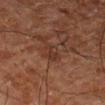Recorded during total-body skin imaging; not selected for excision or biopsy. A male subject aged 78 to 82. Captured under cross-polarized illumination. The lesion is located on the left thigh. The recorded lesion diameter is about 2.5 mm. An algorithmic analysis of the crop reported an area of roughly 3.5 mm² and a shape eccentricity near 0.8. It also reported a lesion color around L≈25 a*≈17 b*≈22 in CIELAB, roughly 5 lightness units darker than nearby skin, and a normalized lesion–skin contrast near 5.5. A region of skin cropped from a whole-body photographic capture, roughly 15 mm wide.